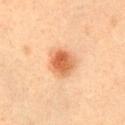illumination: cross-polarized illumination | patient: female, aged 33 to 37 | anatomic site: the left upper arm | image-analysis metrics: an eccentricity of roughly 0.45 and two-axis asymmetry of about 0.15; a lesion color around L≈64 a*≈27 b*≈41 in CIELAB, a lesion–skin lightness drop of about 14, and a lesion-to-skin contrast of about 9 (normalized; higher = more distinct) | size: ~3.5 mm (longest diameter) | image source: ~15 mm crop, total-body skin-cancer survey.